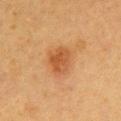biopsy status = imaged on a skin check; not biopsied
body site = the chest
lesion size = ≈3 mm
subject = female, about 40 years old
automated metrics = a lesion color around L≈43 a*≈22 b*≈35 in CIELAB, roughly 8 lightness units darker than nearby skin, and a normalized border contrast of about 7; a border-irregularity index near 2/10 and a within-lesion color-variation index near 3.5/10
tile lighting = cross-polarized
acquisition = total-body-photography crop, ~15 mm field of view